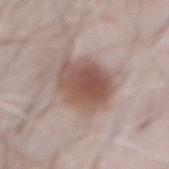{
  "biopsy_status": "not biopsied; imaged during a skin examination",
  "lighting": "white-light",
  "lesion_size": {
    "long_diameter_mm_approx": 5.5
  },
  "patient": {
    "sex": "male",
    "age_approx": 70
  },
  "site": "front of the torso",
  "image": {
    "source": "total-body photography crop",
    "field_of_view_mm": 15
  }
}